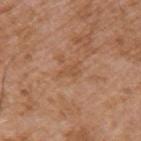biopsy_status: not biopsied; imaged during a skin examination
patient:
  sex: male
  age_approx: 75
image:
  source: total-body photography crop
  field_of_view_mm: 15
site: arm